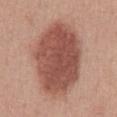Part of a total-body skin-imaging series; this lesion was reviewed on a skin check and was not flagged for biopsy.
From the abdomen.
Measured at roughly 9.5 mm in maximum diameter.
Cropped from a whole-body photographic skin survey; the tile spans about 15 mm.
A male subject aged 53–57.